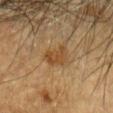{
  "biopsy_status": "not biopsied; imaged during a skin examination",
  "image": {
    "source": "total-body photography crop",
    "field_of_view_mm": 15
  },
  "lighting": "cross-polarized",
  "site": "head or neck",
  "patient": {
    "sex": "male",
    "age_approx": "85+"
  },
  "lesion_size": {
    "long_diameter_mm_approx": 3.5
  }
}